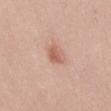The lesion was tiled from a total-body skin photograph and was not biopsied. Approximately 2.5 mm at its widest. A male patient aged around 50. The lesion-visualizer software estimated about 10 CIELAB-L* units darker than the surrounding skin and a lesion-to-skin contrast of about 7 (normalized; higher = more distinct). From the front of the torso. A lesion tile, about 15 mm wide, cut from a 3D total-body photograph.